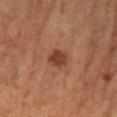Clinical impression:
Recorded during total-body skin imaging; not selected for excision or biopsy.
Context:
From the left lower leg. A roughly 15 mm field-of-view crop from a total-body skin photograph. Measured at roughly 2.5 mm in maximum diameter. The patient is a female roughly 65 years of age.